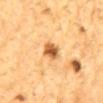From the mid back. A male patient, aged 83 or over. The lesion-visualizer software estimated a footprint of about 5.5 mm² and a symmetry-axis asymmetry near 0.25. And it measured a lesion color around L≈54 a*≈22 b*≈41 in CIELAB, about 15 CIELAB-L* units darker than the surrounding skin, and a normalized border contrast of about 9.5. The analysis additionally found a within-lesion color-variation index near 5/10 and a peripheral color-asymmetry measure near 1.5. Approximately 3 mm at its widest. Captured under cross-polarized illumination. A 15 mm crop from a total-body photograph taken for skin-cancer surveillance.